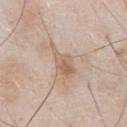The recorded lesion diameter is about 4.5 mm. The subject is a male in their mid-50s. This is a white-light tile. The lesion is on the front of the torso. Cropped from a whole-body photographic skin survey; the tile spans about 15 mm.